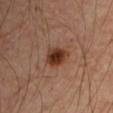Assessment:
Captured during whole-body skin photography for melanoma surveillance; the lesion was not biopsied.
Acquisition and patient details:
The lesion is on the arm. The tile uses cross-polarized illumination. A 15 mm close-up extracted from a 3D total-body photography capture. Automated image analysis of the tile measured a footprint of about 6.5 mm², a shape eccentricity near 0.45, and a symmetry-axis asymmetry near 0.2. The software also gave a border-irregularity rating of about 2/10, a within-lesion color-variation index near 4.5/10, and peripheral color asymmetry of about 1.5. The software also gave a nevus-likeness score of about 100/100 and a detector confidence of about 100 out of 100 that the crop contains a lesion. A male patient, in their mid-60s.